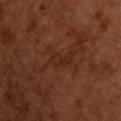The lesion was tiled from a total-body skin photograph and was not biopsied.